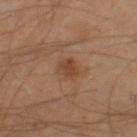Captured during whole-body skin photography for melanoma surveillance; the lesion was not biopsied. Automated tile analysis of the lesion measured a lesion color around L≈42 a*≈20 b*≈31 in CIELAB, about 8 CIELAB-L* units darker than the surrounding skin, and a normalized border contrast of about 7. The software also gave an automated nevus-likeness rating near 45 out of 100. The lesion is on the right thigh. A roughly 15 mm field-of-view crop from a total-body skin photograph. The tile uses cross-polarized illumination. A male patient aged 43 to 47.